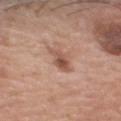notes: total-body-photography surveillance lesion; no biopsy
patient: male, aged around 60
lesion diameter: ~3 mm (longest diameter)
imaging modality: ~15 mm crop, total-body skin-cancer survey
body site: the head or neck
lighting: white-light